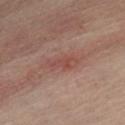| field | value |
|---|---|
| follow-up | imaged on a skin check; not biopsied |
| tile lighting | cross-polarized illumination |
| automated metrics | an area of roughly 7 mm², an outline eccentricity of about 0.9 (0 = round, 1 = elongated), and a shape-asymmetry score of about 0.2 (0 = symmetric); an average lesion color of about L≈39 a*≈19 b*≈21 (CIELAB), a lesion–skin lightness drop of about 6, and a normalized lesion–skin contrast near 5; border irregularity of about 2.5 on a 0–10 scale and radial color variation of about 1 |
| location | the mid back |
| lesion size | ~4 mm (longest diameter) |
| image source | ~15 mm crop, total-body skin-cancer survey |
| patient | female, aged 53 to 57 |